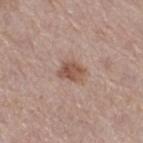Captured during whole-body skin photography for melanoma surveillance; the lesion was not biopsied.